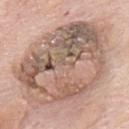notes: imaged on a skin check; not biopsied
illumination: white-light illumination
patient: male, roughly 75 years of age
location: the mid back
size: ≈11.5 mm
image source: total-body-photography crop, ~15 mm field of view